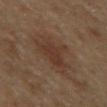Recorded during total-body skin imaging; not selected for excision or biopsy. A roughly 15 mm field-of-view crop from a total-body skin photograph. Located on the abdomen. The patient is a male aged around 65.